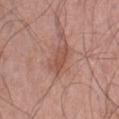  biopsy_status: not biopsied; imaged during a skin examination
  lighting: white-light
  patient:
    sex: male
    age_approx: 70
  site: abdomen
  lesion_size:
    long_diameter_mm_approx: 4.0
  image:
    source: total-body photography crop
    field_of_view_mm: 15